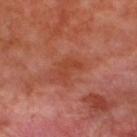Assessment:
Captured during whole-body skin photography for melanoma surveillance; the lesion was not biopsied.
Image and clinical context:
The total-body-photography lesion software estimated an area of roughly 4.5 mm², a shape eccentricity near 0.85, and a shape-asymmetry score of about 0.5 (0 = symmetric). The software also gave an average lesion color of about L≈43 a*≈30 b*≈34 (CIELAB), about 6 CIELAB-L* units darker than the surrounding skin, and a normalized border contrast of about 6. The analysis additionally found a classifier nevus-likeness of about 0/100 and a detector confidence of about 100 out of 100 that the crop contains a lesion. From the upper back. Cropped from a whole-body photographic skin survey; the tile spans about 15 mm. The tile uses cross-polarized illumination. The recorded lesion diameter is about 3.5 mm. A male subject about 50 years old.